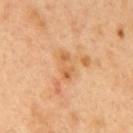Findings:
• notes — catalogued during a skin exam; not biopsied
• body site — the mid back
• subject — male, in their 50s
• diameter — ≈3.5 mm
• image — ~15 mm tile from a whole-body skin photo
• illumination — cross-polarized